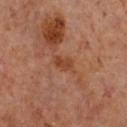The lesion was photographed on a routine skin check and not biopsied; there is no pathology result. A female subject about 60 years old. A 15 mm crop from a total-body photograph taken for skin-cancer surveillance. On the chest. About 2.5 mm across. Captured under cross-polarized illumination.